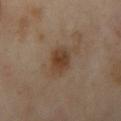Recorded during total-body skin imaging; not selected for excision or biopsy. Cropped from a total-body skin-imaging series; the visible field is about 15 mm. Approximately 3 mm at its widest. Located on the left arm. The patient is a female aged 38–42.